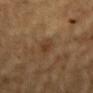Assessment:
Imaged during a routine full-body skin examination; the lesion was not biopsied and no histopathology is available.
Context:
Approximately 2.5 mm at its widest. Located on the mid back. The lesion-visualizer software estimated a lesion area of about 3.5 mm² and a shape-asymmetry score of about 0.2 (0 = symmetric). The software also gave a lesion color around L≈32 a*≈15 b*≈27 in CIELAB, a lesion–skin lightness drop of about 5, and a normalized lesion–skin contrast near 6. A lesion tile, about 15 mm wide, cut from a 3D total-body photograph. A male patient, about 85 years old or older.